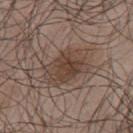The lesion was photographed on a routine skin check and not biopsied; there is no pathology result.
The total-body-photography lesion software estimated a border-irregularity rating of about 3/10 and peripheral color asymmetry of about 1.5. The software also gave a classifier nevus-likeness of about 65/100.
Imaged with white-light lighting.
A close-up tile cropped from a whole-body skin photograph, about 15 mm across.
A male subject, about 50 years old.
From the upper back.
About 6 mm across.